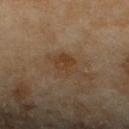Automated tile analysis of the lesion measured a lesion area of about 5.5 mm², an eccentricity of roughly 0.6, and a symmetry-axis asymmetry near 0.3. It also reported a lesion color around L≈35 a*≈15 b*≈28 in CIELAB and a normalized lesion–skin contrast near 6. The software also gave a border-irregularity index near 3/10, a color-variation rating of about 3/10, and peripheral color asymmetry of about 1. The analysis additionally found a classifier nevus-likeness of about 5/100 and a lesion-detection confidence of about 100/100.
From the left lower leg.
A 15 mm close-up extracted from a 3D total-body photography capture.
A female patient roughly 60 years of age.
The lesion's longest dimension is about 3 mm.